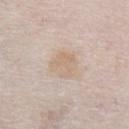Clinical impression: No biopsy was performed on this lesion — it was imaged during a full skin examination and was not determined to be concerning. Background: This is a white-light tile. The subject is a male aged approximately 75. Approximately 3.5 mm at its widest. A region of skin cropped from a whole-body photographic capture, roughly 15 mm wide. The lesion is located on the abdomen. An algorithmic analysis of the crop reported an area of roughly 8 mm² and a symmetry-axis asymmetry near 0.2. It also reported an automated nevus-likeness rating near 10 out of 100 and a detector confidence of about 100 out of 100 that the crop contains a lesion.